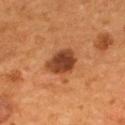workup: total-body-photography surveillance lesion; no biopsy
patient: male, about 55 years old
diameter: about 4 mm
acquisition: ~15 mm tile from a whole-body skin photo
site: the back
tile lighting: cross-polarized
image-analysis metrics: a mean CIELAB color near L≈39 a*≈24 b*≈33; a within-lesion color-variation index near 4/10 and a peripheral color-asymmetry measure near 1